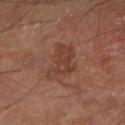Part of a total-body skin-imaging series; this lesion was reviewed on a skin check and was not flagged for biopsy.
Longest diameter approximately 4.5 mm.
This is a cross-polarized tile.
An algorithmic analysis of the crop reported a mean CIELAB color near L≈39 a*≈22 b*≈27 and a lesion-to-skin contrast of about 6 (normalized; higher = more distinct). And it measured border irregularity of about 5 on a 0–10 scale and radial color variation of about 1.
A region of skin cropped from a whole-body photographic capture, roughly 15 mm wide.
On the left lower leg.
The patient is a male aged 68 to 72.